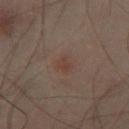{
  "biopsy_status": "not biopsied; imaged during a skin examination",
  "patient": {
    "sex": "male",
    "age_approx": 50
  },
  "site": "leg",
  "lesion_size": {
    "long_diameter_mm_approx": 2.5
  },
  "lighting": "cross-polarized",
  "image": {
    "source": "total-body photography crop",
    "field_of_view_mm": 15
  }
}